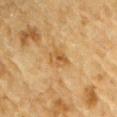Q: Was this lesion biopsied?
A: imaged on a skin check; not biopsied
Q: What is the imaging modality?
A: ~15 mm tile from a whole-body skin photo
Q: What is the lesion's diameter?
A: ~3 mm (longest diameter)
Q: What are the patient's age and sex?
A: male, in their mid- to late 80s or older
Q: What lighting was used for the tile?
A: cross-polarized illumination
Q: Lesion location?
A: the right upper arm
Q: Automated lesion metrics?
A: a lesion area of about 4.5 mm² and an eccentricity of roughly 0.8; an average lesion color of about L≈49 a*≈17 b*≈38 (CIELAB), about 7 CIELAB-L* units darker than the surrounding skin, and a lesion-to-skin contrast of about 6 (normalized; higher = more distinct)